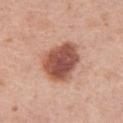Q: Was this lesion biopsied?
A: total-body-photography surveillance lesion; no biopsy
Q: How was this image acquired?
A: ~15 mm tile from a whole-body skin photo
Q: What lighting was used for the tile?
A: white-light illumination
Q: What is the lesion's diameter?
A: about 5.5 mm
Q: What are the patient's age and sex?
A: female, aged around 40
Q: Lesion location?
A: the right thigh
Q: Automated lesion metrics?
A: a color-variation rating of about 5/10 and radial color variation of about 1.5; an automated nevus-likeness rating near 95 out of 100 and a lesion-detection confidence of about 100/100